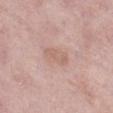biopsy_status: not biopsied; imaged during a skin examination
patient:
  sex: female
  age_approx: 70
lighting: white-light
site: right lower leg
image:
  source: total-body photography crop
  field_of_view_mm: 15
lesion_size:
  long_diameter_mm_approx: 3.5